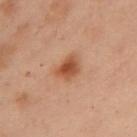Q: Was this lesion biopsied?
A: imaged on a skin check; not biopsied
Q: How large is the lesion?
A: ~3 mm (longest diameter)
Q: Where on the body is the lesion?
A: the chest
Q: Patient demographics?
A: female, about 55 years old
Q: What kind of image is this?
A: ~15 mm crop, total-body skin-cancer survey